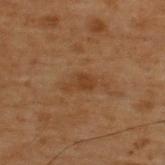The lesion is on the upper back.
Cropped from a total-body skin-imaging series; the visible field is about 15 mm.
The subject is a male aged 68 to 72.
An algorithmic analysis of the crop reported an area of roughly 3.5 mm² and a symmetry-axis asymmetry near 0.4. And it measured a mean CIELAB color near L≈29 a*≈16 b*≈27, about 5 CIELAB-L* units darker than the surrounding skin, and a normalized lesion–skin contrast near 6. The software also gave a classifier nevus-likeness of about 0/100 and lesion-presence confidence of about 100/100.
The tile uses cross-polarized illumination.
Approximately 3 mm at its widest.